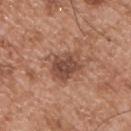No biopsy was performed on this lesion — it was imaged during a full skin examination and was not determined to be concerning. A 15 mm close-up extracted from a 3D total-body photography capture. This is a white-light tile. Longest diameter approximately 4.5 mm. A male subject aged approximately 55. Located on the chest.